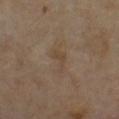Q: Was a biopsy performed?
A: catalogued during a skin exam; not biopsied
Q: Where on the body is the lesion?
A: the chest
Q: How large is the lesion?
A: ≈3.5 mm
Q: Patient demographics?
A: male, aged 68–72
Q: What kind of image is this?
A: ~15 mm crop, total-body skin-cancer survey